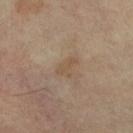follow-up — no biopsy performed (imaged during a skin exam) | image — 15 mm crop, total-body photography | lighting — cross-polarized illumination | automated metrics — a lesion area of about 3 mm²; a border-irregularity index near 3/10 and a color-variation rating of about 0/10; a classifier nevus-likeness of about 0/100 | lesion diameter — ~3 mm (longest diameter) | anatomic site — the right lower leg | subject — female, in their mid- to late 60s.